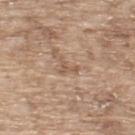{
  "biopsy_status": "not biopsied; imaged during a skin examination",
  "lighting": "white-light",
  "patient": {
    "sex": "male",
    "age_approx": 65
  },
  "image": {
    "source": "total-body photography crop",
    "field_of_view_mm": 15
  },
  "automated_metrics": {
    "border_irregularity_0_10": 8.0,
    "color_variation_0_10": 0.0,
    "peripheral_color_asymmetry": 0.0,
    "nevus_likeness_0_100": 0,
    "lesion_detection_confidence_0_100": 50
  },
  "lesion_size": {
    "long_diameter_mm_approx": 2.5
  },
  "site": "upper back"
}